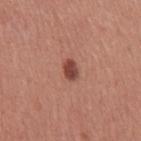Notes:
– follow-up: catalogued during a skin exam; not biopsied